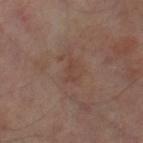<record>
  <biopsy_status>not biopsied; imaged during a skin examination</biopsy_status>
  <patient>
    <sex>male</sex>
    <age_approx>65</age_approx>
  </patient>
  <lesion_size>
    <long_diameter_mm_approx>2.5</long_diameter_mm_approx>
  </lesion_size>
  <lighting>cross-polarized</lighting>
  <site>right thigh</site>
  <automated_metrics>
    <area_mm2_approx>2.0</area_mm2_approx>
    <eccentricity>0.9</eccentricity>
    <shape_asymmetry>0.45</shape_asymmetry>
    <border_irregularity_0_10>5.5</border_irregularity_0_10>
    <peripheral_color_asymmetry>0.0</peripheral_color_asymmetry>
    <lesion_detection_confidence_0_100>100</lesion_detection_confidence_0_100>
  </automated_metrics>
  <image>
    <source>total-body photography crop</source>
    <field_of_view_mm>15</field_of_view_mm>
  </image>
</record>